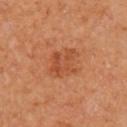Part of a total-body skin-imaging series; this lesion was reviewed on a skin check and was not flagged for biopsy. Longest diameter approximately 4.5 mm. The tile uses cross-polarized illumination. This image is a 15 mm lesion crop taken from a total-body photograph. An algorithmic analysis of the crop reported an area of roughly 12 mm², an outline eccentricity of about 0.6 (0 = round, 1 = elongated), and two-axis asymmetry of about 0.2. The software also gave a border-irregularity rating of about 2.5/10, internal color variation of about 4 on a 0–10 scale, and a peripheral color-asymmetry measure near 1. And it measured a detector confidence of about 100 out of 100 that the crop contains a lesion. On the upper back. A female patient.